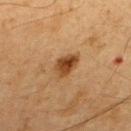Background: This is a cross-polarized tile. A lesion tile, about 15 mm wide, cut from a 3D total-body photograph. A male subject about 65 years old. The recorded lesion diameter is about 3 mm. Located on the upper back.